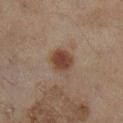Q: Was this lesion biopsied?
A: imaged on a skin check; not biopsied
Q: Automated lesion metrics?
A: a lesion area of about 7.5 mm², a shape eccentricity near 0.45, and a symmetry-axis asymmetry near 0.15; roughly 10 lightness units darker than nearby skin and a lesion-to-skin contrast of about 9 (normalized; higher = more distinct)
Q: How large is the lesion?
A: ≈3 mm
Q: Who is the patient?
A: female, aged around 60
Q: How was this image acquired?
A: 15 mm crop, total-body photography
Q: What lighting was used for the tile?
A: cross-polarized
Q: Where on the body is the lesion?
A: the right lower leg